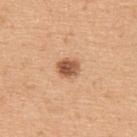No biopsy was performed on this lesion — it was imaged during a full skin examination and was not determined to be concerning. A male patient, aged approximately 50. From the upper back. A close-up tile cropped from a whole-body skin photograph, about 15 mm across.